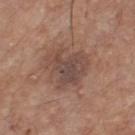notes=imaged on a skin check; not biopsied | image source=~15 mm tile from a whole-body skin photo | subject=male, roughly 75 years of age | site=the chest | tile lighting=white-light | diameter=≈4.5 mm.